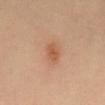Part of a total-body skin-imaging series; this lesion was reviewed on a skin check and was not flagged for biopsy.
The tile uses cross-polarized illumination.
The lesion is located on the mid back.
A region of skin cropped from a whole-body photographic capture, roughly 15 mm wide.
Automated image analysis of the tile measured a within-lesion color-variation index near 2/10.
Measured at roughly 2.5 mm in maximum diameter.
A female subject aged 38–42.